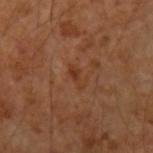Clinical impression: The lesion was photographed on a routine skin check and not biopsied; there is no pathology result. Background: A male patient, aged around 55. Automated tile analysis of the lesion measured a mean CIELAB color near L≈37 a*≈23 b*≈33, roughly 6 lightness units darker than nearby skin, and a normalized lesion–skin contrast near 6. It also reported border irregularity of about 4 on a 0–10 scale, a color-variation rating of about 0/10, and radial color variation of about 0. It also reported a nevus-likeness score of about 0/100 and lesion-presence confidence of about 100/100. A close-up tile cropped from a whole-body skin photograph, about 15 mm across. On the left forearm. The tile uses cross-polarized illumination. Measured at roughly 2.5 mm in maximum diameter.